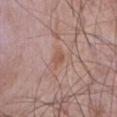notes=no biopsy performed (imaged during a skin exam)
acquisition=total-body-photography crop, ~15 mm field of view
site=the abdomen
lesion size=about 2.5 mm
patient=male, in their mid-60s